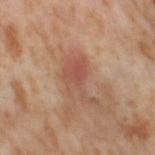Captured under cross-polarized illumination. From the right thigh. A lesion tile, about 15 mm wide, cut from a 3D total-body photograph. A female subject in their mid-50s. The recorded lesion diameter is about 5.5 mm.